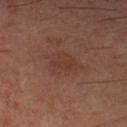Q: Is there a histopathology result?
A: no biopsy performed (imaged during a skin exam)
Q: Where on the body is the lesion?
A: the leg
Q: What lighting was used for the tile?
A: cross-polarized
Q: How large is the lesion?
A: ≈4 mm
Q: What did automated image analysis measure?
A: a lesion area of about 9 mm², a shape eccentricity near 0.7, and a shape-asymmetry score of about 0.25 (0 = symmetric); a mean CIELAB color near L≈34 a*≈19 b*≈24, about 5 CIELAB-L* units darker than the surrounding skin, and a normalized border contrast of about 5; border irregularity of about 3 on a 0–10 scale, internal color variation of about 1.5 on a 0–10 scale, and radial color variation of about 0.5; an automated nevus-likeness rating near 0 out of 100
Q: What is the imaging modality?
A: ~15 mm tile from a whole-body skin photo
Q: Patient demographics?
A: male, about 60 years old